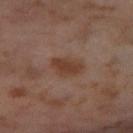The lesion was tiled from a total-body skin photograph and was not biopsied. Imaged with cross-polarized lighting. Approximately 3.5 mm at its widest. Cropped from a total-body skin-imaging series; the visible field is about 15 mm. A female subject aged 53 to 57. The lesion is located on the right thigh. The total-body-photography lesion software estimated a mean CIELAB color near L≈39 a*≈20 b*≈28, about 8 CIELAB-L* units darker than the surrounding skin, and a normalized lesion–skin contrast near 8.5. The software also gave a nevus-likeness score of about 90/100 and a detector confidence of about 100 out of 100 that the crop contains a lesion.